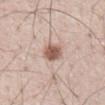Findings:
– anatomic site — the mid back
– subject — male, in their 50s
– diameter — ~3.5 mm (longest diameter)
– illumination — white-light illumination
– imaging modality — 15 mm crop, total-body photography